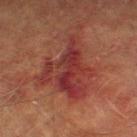biopsy status = no biopsy performed (imaged during a skin exam); image = ~15 mm tile from a whole-body skin photo; tile lighting = cross-polarized illumination; patient = male, approximately 75 years of age; site = the left lower leg.